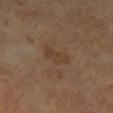Clinical summary: Imaged with cross-polarized lighting. From the left lower leg. Longest diameter approximately 3.5 mm. A 15 mm close-up tile from a total-body photography series done for melanoma screening. The patient is a male in their mid-60s.